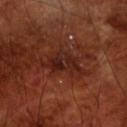Assessment: This lesion was catalogued during total-body skin photography and was not selected for biopsy. Context: A 15 mm crop from a total-body photograph taken for skin-cancer surveillance. Automated image analysis of the tile measured a nevus-likeness score of about 0/100 and a lesion-detection confidence of about 100/100. On the front of the torso. The subject is a male approximately 70 years of age.